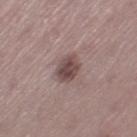The lesion was photographed on a routine skin check and not biopsied; there is no pathology result.
Longest diameter approximately 4 mm.
A 15 mm close-up tile from a total-body photography series done for melanoma screening.
The lesion is on the left thigh.
Automated tile analysis of the lesion measured a lesion area of about 7 mm² and a shape eccentricity near 0.75. It also reported roughly 12 lightness units darker than nearby skin and a lesion-to-skin contrast of about 9 (normalized; higher = more distinct). It also reported a border-irregularity rating of about 2/10, a within-lesion color-variation index near 3.5/10, and a peripheral color-asymmetry measure near 1.
A female subject aged around 45.